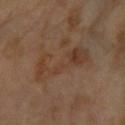follow-up: imaged on a skin check; not biopsied | patient: female, roughly 70 years of age | tile lighting: cross-polarized | automated lesion analysis: an average lesion color of about L≈39 a*≈18 b*≈29 (CIELAB) and a normalized lesion–skin contrast near 6; a border-irregularity index near 9/10 and a color-variation rating of about 3.5/10 | anatomic site: the right forearm | acquisition: 15 mm crop, total-body photography | size: ≈7.5 mm.